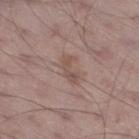follow-up: imaged on a skin check; not biopsied
lighting: white-light
automated metrics: a mean CIELAB color near L≈51 a*≈17 b*≈23, roughly 7 lightness units darker than nearby skin, and a lesion-to-skin contrast of about 5.5 (normalized; higher = more distinct); border irregularity of about 6 on a 0–10 scale, internal color variation of about 0 on a 0–10 scale, and radial color variation of about 0; a classifier nevus-likeness of about 0/100 and a lesion-detection confidence of about 100/100
body site: the leg
lesion size: about 3 mm
subject: male, roughly 65 years of age
image source: total-body-photography crop, ~15 mm field of view